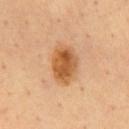No biopsy was performed on this lesion — it was imaged during a full skin examination and was not determined to be concerning. A lesion tile, about 15 mm wide, cut from a 3D total-body photograph. The lesion is located on the front of the torso. Captured under cross-polarized illumination. The patient is a male aged 63 to 67. The total-body-photography lesion software estimated an eccentricity of roughly 0.75 and two-axis asymmetry of about 0.15. And it measured a mean CIELAB color near L≈55 a*≈23 b*≈41, roughly 13 lightness units darker than nearby skin, and a lesion-to-skin contrast of about 9.5 (normalized; higher = more distinct). And it measured a border-irregularity rating of about 2/10 and radial color variation of about 2. The lesion's longest dimension is about 4.5 mm.